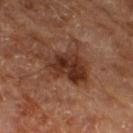The lesion was tiled from a total-body skin photograph and was not biopsied.
A subject in their mid-60s.
This is a cross-polarized tile.
Approximately 6.5 mm at its widest.
On the right thigh.
A close-up tile cropped from a whole-body skin photograph, about 15 mm across.